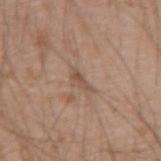| field | value |
|---|---|
| subject | male, aged 18 to 22 |
| anatomic site | the right upper arm |
| image source | 15 mm crop, total-body photography |